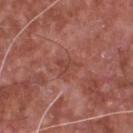Background: A male patient about 65 years old. Located on the chest. Longest diameter approximately 3 mm. A 15 mm crop from a total-body photograph taken for skin-cancer surveillance.